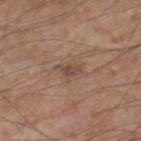Assessment: This lesion was catalogued during total-body skin photography and was not selected for biopsy. Context: A male subject approximately 55 years of age. A 15 mm close-up tile from a total-body photography series done for melanoma screening. An algorithmic analysis of the crop reported a lesion area of about 3.5 mm², a shape eccentricity near 0.7, and a shape-asymmetry score of about 0.4 (0 = symmetric). It also reported a lesion color around L≈47 a*≈18 b*≈25 in CIELAB, about 8 CIELAB-L* units darker than the surrounding skin, and a normalized lesion–skin contrast near 6. It also reported a detector confidence of about 100 out of 100 that the crop contains a lesion. The lesion is located on the right lower leg.